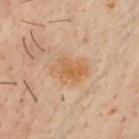follow-up: catalogued during a skin exam; not biopsied | subject: male, aged 48 to 52 | acquisition: ~15 mm crop, total-body skin-cancer survey | lesion size: about 4.5 mm | body site: the chest.